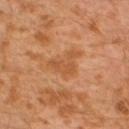<tbp_lesion>
  <biopsy_status>not biopsied; imaged during a skin examination</biopsy_status>
  <site>left lower leg</site>
  <image>
    <source>total-body photography crop</source>
    <field_of_view_mm>15</field_of_view_mm>
  </image>
  <lighting>cross-polarized</lighting>
  <lesion_size>
    <long_diameter_mm_approx>4.5</long_diameter_mm_approx>
  </lesion_size>
  <patient>
    <sex>male</sex>
    <age_approx>30</age_approx>
  </patient>
</tbp_lesion>